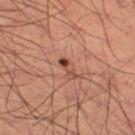Q: Was a biopsy performed?
A: catalogued during a skin exam; not biopsied
Q: What lighting was used for the tile?
A: cross-polarized illumination
Q: Lesion location?
A: the right thigh
Q: How large is the lesion?
A: ~3 mm (longest diameter)
Q: What is the imaging modality?
A: total-body-photography crop, ~15 mm field of view
Q: What are the patient's age and sex?
A: male, aged around 50